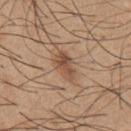Impression: The lesion was photographed on a routine skin check and not biopsied; there is no pathology result. Clinical summary: A male patient approximately 65 years of age. From the chest. Cropped from a total-body skin-imaging series; the visible field is about 15 mm. The lesion-visualizer software estimated a mean CIELAB color near L≈50 a*≈19 b*≈30, about 10 CIELAB-L* units darker than the surrounding skin, and a lesion-to-skin contrast of about 7 (normalized; higher = more distinct). It also reported an automated nevus-likeness rating near 80 out of 100 and a detector confidence of about 100 out of 100 that the crop contains a lesion. The tile uses white-light illumination.